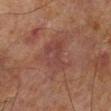<lesion>
  <biopsy_status>not biopsied; imaged during a skin examination</biopsy_status>
  <lesion_size>
    <long_diameter_mm_approx>4.5</long_diameter_mm_approx>
  </lesion_size>
  <site>leg</site>
  <image>
    <source>total-body photography crop</source>
    <field_of_view_mm>15</field_of_view_mm>
  </image>
  <patient>
    <sex>male</sex>
    <age_approx>70</age_approx>
  </patient>
  <lighting>cross-polarized</lighting>
</lesion>